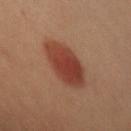{
  "image": {
    "source": "total-body photography crop",
    "field_of_view_mm": 15
  },
  "patient": {
    "sex": "female",
    "age_approx": 40
  },
  "site": "left arm",
  "lighting": "cross-polarized",
  "automated_metrics": {
    "area_mm2_approx": 17.0,
    "eccentricity": 0.8,
    "shape_asymmetry": 0.1,
    "nevus_likeness_0_100": 100,
    "lesion_detection_confidence_0_100": 100
  },
  "lesion_size": {
    "long_diameter_mm_approx": 6.0
  }
}